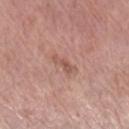Clinical impression:
The lesion was tiled from a total-body skin photograph and was not biopsied.
Clinical summary:
A roughly 15 mm field-of-view crop from a total-body skin photograph. The patient is a male approximately 60 years of age. From the left forearm. The lesion-visualizer software estimated a border-irregularity index near 4.5/10, a color-variation rating of about 0/10, and a peripheral color-asymmetry measure near 0. Longest diameter approximately 3 mm. This is a white-light tile.